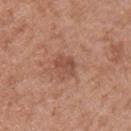Background: From the right upper arm. A female subject, roughly 40 years of age. Cropped from a total-body skin-imaging series; the visible field is about 15 mm.